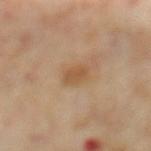Q: Was a biopsy performed?
A: total-body-photography surveillance lesion; no biopsy
Q: What are the patient's age and sex?
A: male, aged 68–72
Q: How was this image acquired?
A: ~15 mm tile from a whole-body skin photo
Q: Illumination type?
A: cross-polarized illumination
Q: What did automated image analysis measure?
A: an area of roughly 4 mm² and an outline eccentricity of about 0.6 (0 = round, 1 = elongated); an average lesion color of about L≈51 a*≈18 b*≈34 (CIELAB)
Q: How large is the lesion?
A: ~2.5 mm (longest diameter)
Q: Where on the body is the lesion?
A: the mid back